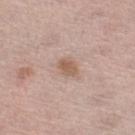Impression: Imaged during a routine full-body skin examination; the lesion was not biopsied and no histopathology is available. Acquisition and patient details: Located on the left lower leg. Cropped from a whole-body photographic skin survey; the tile spans about 15 mm. An algorithmic analysis of the crop reported a lesion-detection confidence of about 100/100. A female subject aged 63–67.